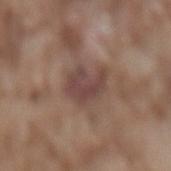* notes · imaged on a skin check; not biopsied
* lesion size · ≈4 mm
* image · ~15 mm crop, total-body skin-cancer survey
* body site · the back
* patient · male, aged approximately 75
* TBP lesion metrics · an average lesion color of about L≈43 a*≈18 b*≈21 (CIELAB), about 9 CIELAB-L* units darker than the surrounding skin, and a lesion-to-skin contrast of about 7.5 (normalized; higher = more distinct); a border-irregularity rating of about 3.5/10, internal color variation of about 3.5 on a 0–10 scale, and radial color variation of about 1; lesion-presence confidence of about 100/100
* illumination · white-light illumination